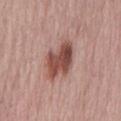Imaged during a routine full-body skin examination; the lesion was not biopsied and no histopathology is available.
Captured under white-light illumination.
From the mid back.
The subject is a male in their mid-60s.
The total-body-photography lesion software estimated a lesion color around L≈49 a*≈23 b*≈25 in CIELAB, about 14 CIELAB-L* units darker than the surrounding skin, and a normalized lesion–skin contrast near 10.
A 15 mm crop from a total-body photograph taken for skin-cancer surveillance.